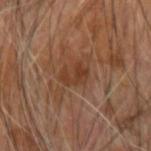This lesion was catalogued during total-body skin photography and was not selected for biopsy.
A region of skin cropped from a whole-body photographic capture, roughly 15 mm wide.
From the right forearm.
A male subject, aged approximately 65.
Approximately 3 mm at its widest.